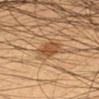The lesion was photographed on a routine skin check and not biopsied; there is no pathology result. Automated tile analysis of the lesion measured a footprint of about 5.5 mm² and two-axis asymmetry of about 0.25. The software also gave an average lesion color of about L≈45 a*≈20 b*≈33 (CIELAB) and a lesion-to-skin contrast of about 7.5 (normalized; higher = more distinct). The analysis additionally found an automated nevus-likeness rating near 95 out of 100 and a lesion-detection confidence of about 100/100. On the left forearm. A male subject aged 33–37. Approximately 3 mm at its widest. A region of skin cropped from a whole-body photographic capture, roughly 15 mm wide. The tile uses cross-polarized illumination.